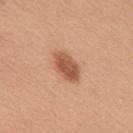Case summary:
• follow-up · catalogued during a skin exam; not biopsied
• lighting · white-light illumination
• patient · female, in their mid-40s
• site · the upper back
• imaging modality · ~15 mm crop, total-body skin-cancer survey
• automated lesion analysis · a shape eccentricity near 0.8 and a shape-asymmetry score of about 0.2 (0 = symmetric); an average lesion color of about L≈55 a*≈24 b*≈34 (CIELAB), about 13 CIELAB-L* units darker than the surrounding skin, and a lesion-to-skin contrast of about 8.5 (normalized; higher = more distinct); lesion-presence confidence of about 100/100